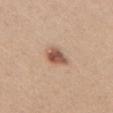Q: Was this lesion biopsied?
A: no biopsy performed (imaged during a skin exam)
Q: Who is the patient?
A: female, roughly 45 years of age
Q: What is the anatomic site?
A: the chest
Q: What is the lesion's diameter?
A: ≈3 mm
Q: How was the tile lit?
A: white-light illumination
Q: How was this image acquired?
A: ~15 mm tile from a whole-body skin photo